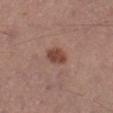Clinical impression: Imaged during a routine full-body skin examination; the lesion was not biopsied and no histopathology is available. Context: An algorithmic analysis of the crop reported a lesion–skin lightness drop of about 11. And it measured a classifier nevus-likeness of about 90/100 and a detector confidence of about 100 out of 100 that the crop contains a lesion. A lesion tile, about 15 mm wide, cut from a 3D total-body photograph. The recorded lesion diameter is about 2.5 mm. The lesion is on the right lower leg. The tile uses white-light illumination. The subject is a male aged approximately 55.